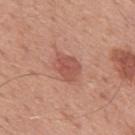The lesion was tiled from a total-body skin photograph and was not biopsied. A male patient aged approximately 50. This is a white-light tile. A close-up tile cropped from a whole-body skin photograph, about 15 mm across. The lesion is on the mid back.